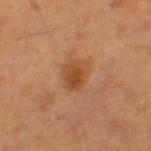| feature | finding |
|---|---|
| workup | imaged on a skin check; not biopsied |
| acquisition | ~15 mm tile from a whole-body skin photo |
| lesion diameter | ~3.5 mm (longest diameter) |
| subject | female, approximately 55 years of age |
| tile lighting | cross-polarized illumination |
| site | the left thigh |